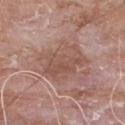follow-up: total-body-photography surveillance lesion; no biopsy
patient: male, aged around 55
acquisition: total-body-photography crop, ~15 mm field of view
tile lighting: white-light illumination
location: the chest
TBP lesion metrics: a lesion color around L≈52 a*≈20 b*≈25 in CIELAB, about 8 CIELAB-L* units darker than the surrounding skin, and a normalized lesion–skin contrast near 6; a border-irregularity index near 4.5/10 and internal color variation of about 4 on a 0–10 scale; a classifier nevus-likeness of about 0/100 and a lesion-detection confidence of about 100/100